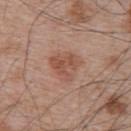notes: imaged on a skin check; not biopsied
tile lighting: white-light illumination
anatomic site: the upper back
automated lesion analysis: a lesion color around L≈52 a*≈22 b*≈29 in CIELAB, roughly 8 lightness units darker than nearby skin, and a normalized lesion–skin contrast near 6.5; a nevus-likeness score of about 20/100
patient: male, aged 63–67
image: ~15 mm tile from a whole-body skin photo
diameter: ≈4 mm